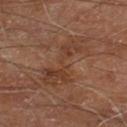| key | value |
|---|---|
| workup | total-body-photography surveillance lesion; no biopsy |
| location | the right lower leg |
| acquisition | ~15 mm tile from a whole-body skin photo |
| subject | male, approximately 70 years of age |
| TBP lesion metrics | a lesion area of about 15 mm²; an average lesion color of about L≈38 a*≈20 b*≈28 (CIELAB) and a lesion-to-skin contrast of about 5.5 (normalized; higher = more distinct); an automated nevus-likeness rating near 0 out of 100 and a detector confidence of about 100 out of 100 that the crop contains a lesion |
| lesion diameter | ~8 mm (longest diameter) |